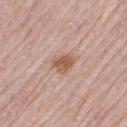Assessment: This lesion was catalogued during total-body skin photography and was not selected for biopsy. Context: On the leg. Automated image analysis of the tile measured a footprint of about 4.5 mm², a shape eccentricity near 0.55, and two-axis asymmetry of about 0.3. The analysis additionally found a border-irregularity index near 2.5/10, a within-lesion color-variation index near 2/10, and radial color variation of about 1. A female patient, about 65 years old. About 2.5 mm across. A 15 mm crop from a total-body photograph taken for skin-cancer surveillance. Imaged with white-light lighting.